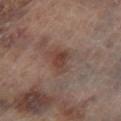Assessment: Captured during whole-body skin photography for melanoma surveillance; the lesion was not biopsied. Background: The tile uses cross-polarized illumination. A male subject, aged 63 to 67. A close-up tile cropped from a whole-body skin photograph, about 15 mm across. The lesion is on the left lower leg. The total-body-photography lesion software estimated an average lesion color of about L≈38 a*≈18 b*≈22 (CIELAB), about 7 CIELAB-L* units darker than the surrounding skin, and a normalized lesion–skin contrast near 7. The analysis additionally found a nevus-likeness score of about 40/100. The recorded lesion diameter is about 3 mm.